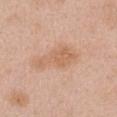No biopsy was performed on this lesion — it was imaged during a full skin examination and was not determined to be concerning.
A 15 mm crop from a total-body photograph taken for skin-cancer surveillance.
The subject is a female in their mid- to late 20s.
The lesion is on the chest.